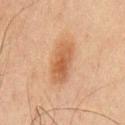Findings:
* notes — catalogued during a skin exam; not biopsied
* image-analysis metrics — an area of roughly 11 mm², an eccentricity of roughly 0.85, and two-axis asymmetry of about 0.2; a color-variation rating of about 3.5/10
* site — the chest
* patient — male, aged 68 to 72
* image source — total-body-photography crop, ~15 mm field of view
* lesion size — ~5 mm (longest diameter)